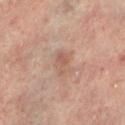| key | value |
|---|---|
| biopsy status | no biopsy performed (imaged during a skin exam) |
| lesion diameter | ≈3 mm |
| subject | male, roughly 65 years of age |
| site | the leg |
| lighting | cross-polarized illumination |
| automated lesion analysis | an average lesion color of about L≈54 a*≈20 b*≈26 (CIELAB), about 7 CIELAB-L* units darker than the surrounding skin, and a normalized border contrast of about 5.5 |
| image | ~15 mm crop, total-body skin-cancer survey |